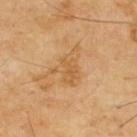{
  "biopsy_status": "not biopsied; imaged during a skin examination"
}